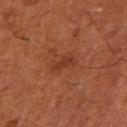The lesion was tiled from a total-body skin photograph and was not biopsied. Captured under cross-polarized illumination. A 15 mm crop from a total-body photograph taken for skin-cancer surveillance. A male patient, approximately 60 years of age. The lesion is located on the left arm. Approximately 3 mm at its widest.